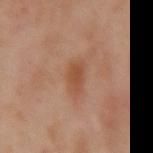Q: Is there a histopathology result?
A: catalogued during a skin exam; not biopsied